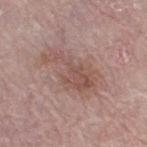Clinical impression: Recorded during total-body skin imaging; not selected for excision or biopsy. Context: A 15 mm close-up tile from a total-body photography series done for melanoma screening. A female patient, about 65 years old. Located on the leg. The lesion-visualizer software estimated a footprint of about 13 mm², an outline eccentricity of about 0.85 (0 = round, 1 = elongated), and a shape-asymmetry score of about 0.5 (0 = symmetric). And it measured a mean CIELAB color near L≈52 a*≈20 b*≈23, about 8 CIELAB-L* units darker than the surrounding skin, and a normalized lesion–skin contrast near 6.5. It also reported a nevus-likeness score of about 0/100 and lesion-presence confidence of about 100/100. This is a white-light tile.